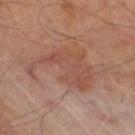biopsy status: imaged on a skin check; not biopsied
acquisition: 15 mm crop, total-body photography
patient: male, about 70 years old
diameter: ≈7.5 mm
lighting: cross-polarized
body site: the right thigh